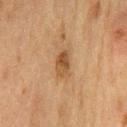The lesion-visualizer software estimated a lesion color around L≈41 a*≈17 b*≈31 in CIELAB and a lesion–skin lightness drop of about 9. And it measured a border-irregularity index near 3.5/10 and a color-variation rating of about 5.5/10. And it measured a nevus-likeness score of about 70/100 and a lesion-detection confidence of about 100/100. The lesion is on the chest. The patient is a male in their mid- to late 70s. Approximately 3.5 mm at its widest. This is a cross-polarized tile. Cropped from a total-body skin-imaging series; the visible field is about 15 mm.